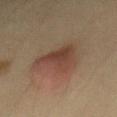{"biopsy_status": "not biopsied; imaged during a skin examination", "patient": {"sex": "male", "age_approx": 40}, "lighting": "cross-polarized", "image": {"source": "total-body photography crop", "field_of_view_mm": 15}, "site": "mid back", "lesion_size": {"long_diameter_mm_approx": 5.0}}